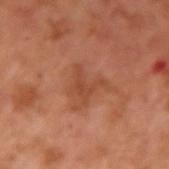follow-up: imaged on a skin check; not biopsied
acquisition: ~15 mm crop, total-body skin-cancer survey
patient: male, aged around 55
site: the left upper arm